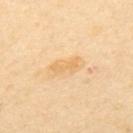Part of a total-body skin-imaging series; this lesion was reviewed on a skin check and was not flagged for biopsy.
A male patient aged 53–57.
The lesion is located on the upper back.
This is a cross-polarized tile.
A 15 mm close-up tile from a total-body photography series done for melanoma screening.